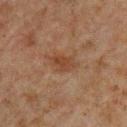Captured during whole-body skin photography for melanoma surveillance; the lesion was not biopsied.
Captured under cross-polarized illumination.
Located on the chest.
A male patient, aged around 60.
A 15 mm close-up extracted from a 3D total-body photography capture.